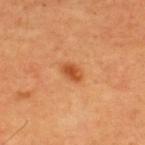follow-up: catalogued during a skin exam; not biopsied | illumination: cross-polarized illumination | size: about 2.5 mm | anatomic site: the upper back | image source: 15 mm crop, total-body photography | automated lesion analysis: an eccentricity of roughly 0.8; a lesion–skin lightness drop of about 11 and a lesion-to-skin contrast of about 8 (normalized; higher = more distinct) | subject: male, aged 63–67.